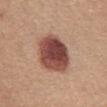Image and clinical context: The total-body-photography lesion software estimated a lesion area of about 20 mm², an outline eccentricity of about 0.6 (0 = round, 1 = elongated), and a shape-asymmetry score of about 0.15 (0 = symmetric). From the abdomen. The subject is a female about 55 years old. This image is a 15 mm lesion crop taken from a total-body photograph.